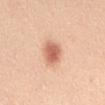Impression: The lesion was tiled from a total-body skin photograph and was not biopsied. Context: The lesion is located on the abdomen. A region of skin cropped from a whole-body photographic capture, roughly 15 mm wide. A female patient about 45 years old.